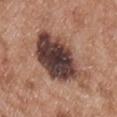The lesion was tiled from a total-body skin photograph and was not biopsied. Longest diameter approximately 8.5 mm. The patient is a male in their mid-50s. On the chest. A region of skin cropped from a whole-body photographic capture, roughly 15 mm wide.